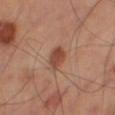Recorded during total-body skin imaging; not selected for excision or biopsy.
Approximately 3 mm at its widest.
The tile uses cross-polarized illumination.
A close-up tile cropped from a whole-body skin photograph, about 15 mm across.
From the right thigh.